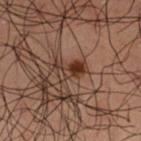Findings:
- biopsy status · catalogued during a skin exam; not biopsied
- anatomic site · the left thigh
- patient · male, in their 50s
- acquisition · ~15 mm crop, total-body skin-cancer survey